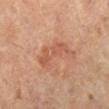Clinical impression:
This lesion was catalogued during total-body skin photography and was not selected for biopsy.
Clinical summary:
A female subject aged 58 to 62. This is a cross-polarized tile. Automated tile analysis of the lesion measured a lesion color around L≈58 a*≈25 b*≈34 in CIELAB, a lesion–skin lightness drop of about 8, and a normalized lesion–skin contrast near 5.5. And it measured peripheral color asymmetry of about 1. And it measured a classifier nevus-likeness of about 10/100 and a detector confidence of about 100 out of 100 that the crop contains a lesion. A 15 mm close-up extracted from a 3D total-body photography capture. The lesion is on the leg.